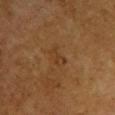Part of a total-body skin-imaging series; this lesion was reviewed on a skin check and was not flagged for biopsy. A female subject, in their mid- to late 50s. From the upper back. A roughly 15 mm field-of-view crop from a total-body skin photograph.